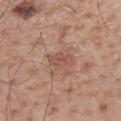Assessment:
The lesion was tiled from a total-body skin photograph and was not biopsied.
Background:
The patient is a male roughly 55 years of age. The lesion is located on the mid back. An algorithmic analysis of the crop reported a lesion color around L≈52 a*≈22 b*≈27 in CIELAB, roughly 8 lightness units darker than nearby skin, and a normalized lesion–skin contrast near 6. The recorded lesion diameter is about 3 mm. Imaged with white-light lighting. Cropped from a total-body skin-imaging series; the visible field is about 15 mm.